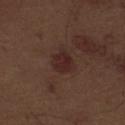Recorded during total-body skin imaging; not selected for excision or biopsy. An algorithmic analysis of the crop reported a lesion area of about 6 mm², an outline eccentricity of about 0.45 (0 = round, 1 = elongated), and a symmetry-axis asymmetry near 0.2. The software also gave a lesion color around L≈25 a*≈19 b*≈19 in CIELAB, a lesion–skin lightness drop of about 7, and a normalized lesion–skin contrast near 8. The software also gave an automated nevus-likeness rating near 40 out of 100 and lesion-presence confidence of about 100/100. On the abdomen. Imaged with white-light lighting. A 15 mm crop from a total-body photograph taken for skin-cancer surveillance. A male subject roughly 70 years of age.